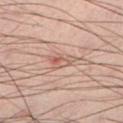{
  "biopsy_status": "not biopsied; imaged during a skin examination"
}